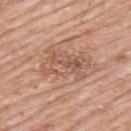notes: total-body-photography surveillance lesion; no biopsy | image source: 15 mm crop, total-body photography | lesion size: about 5 mm | image-analysis metrics: a lesion area of about 12 mm², an outline eccentricity of about 0.8 (0 = round, 1 = elongated), and a symmetry-axis asymmetry near 0.25; a lesion–skin lightness drop of about 8 and a normalized border contrast of about 5.5; a border-irregularity index near 5/10, a within-lesion color-variation index near 6.5/10, and radial color variation of about 2.5 | location: the upper back | tile lighting: white-light | subject: male, roughly 80 years of age.